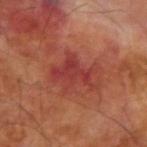Q: Is there a histopathology result?
A: total-body-photography surveillance lesion; no biopsy
Q: What is the imaging modality?
A: ~15 mm tile from a whole-body skin photo
Q: Lesion location?
A: the right upper arm
Q: Illumination type?
A: cross-polarized illumination
Q: Patient demographics?
A: male, aged 68 to 72
Q: Automated lesion metrics?
A: a mean CIELAB color near L≈39 a*≈33 b*≈27, about 8 CIELAB-L* units darker than the surrounding skin, and a normalized border contrast of about 7; internal color variation of about 3 on a 0–10 scale and radial color variation of about 1